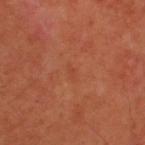The lesion was photographed on a routine skin check and not biopsied; there is no pathology result. A subject aged approximately 55. Imaged with cross-polarized lighting. The lesion's longest dimension is about 2 mm. The lesion is located on the head or neck. A 15 mm crop from a total-body photograph taken for skin-cancer surveillance.